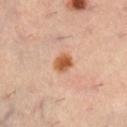The lesion was tiled from a total-body skin photograph and was not biopsied. A female subject in their mid-30s. Imaged with cross-polarized lighting. From the right lower leg. A roughly 15 mm field-of-view crop from a total-body skin photograph. Automated tile analysis of the lesion measured about 13 CIELAB-L* units darker than the surrounding skin and a lesion-to-skin contrast of about 10 (normalized; higher = more distinct). It also reported a border-irregularity index near 1.5/10, a within-lesion color-variation index near 3/10, and radial color variation of about 1. The software also gave a nevus-likeness score of about 95/100 and a detector confidence of about 100 out of 100 that the crop contains a lesion. The lesion's longest dimension is about 2.5 mm.